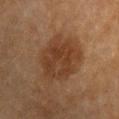Clinical impression: No biopsy was performed on this lesion — it was imaged during a full skin examination and was not determined to be concerning. Context: The patient is a female aged around 55. This is a cross-polarized tile. On the arm. Cropped from a whole-body photographic skin survey; the tile spans about 15 mm.